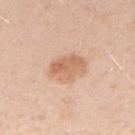Context:
Located on the right forearm. A close-up tile cropped from a whole-body skin photograph, about 15 mm across. A female subject, aged around 20.